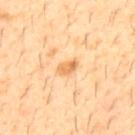Imaged during a routine full-body skin examination; the lesion was not biopsied and no histopathology is available. Longest diameter approximately 2.5 mm. From the upper back. Imaged with cross-polarized lighting. This image is a 15 mm lesion crop taken from a total-body photograph. A male patient, aged approximately 30. The lesion-visualizer software estimated a lesion color around L≈70 a*≈22 b*≈45 in CIELAB. It also reported an automated nevus-likeness rating near 15 out of 100 and a detector confidence of about 100 out of 100 that the crop contains a lesion.